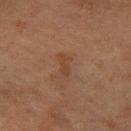biopsy status: no biopsy performed (imaged during a skin exam)
lesion diameter: ~2.5 mm (longest diameter)
automated metrics: a lesion-to-skin contrast of about 5 (normalized; higher = more distinct); a border-irregularity index near 3.5/10, internal color variation of about 0 on a 0–10 scale, and peripheral color asymmetry of about 0; an automated nevus-likeness rating near 0 out of 100
acquisition: total-body-photography crop, ~15 mm field of view
location: the right forearm
illumination: cross-polarized illumination
subject: female, aged 58–62